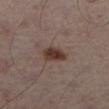Clinical impression: The lesion was photographed on a routine skin check and not biopsied; there is no pathology result. Clinical summary: A roughly 15 mm field-of-view crop from a total-body skin photograph. The lesion's longest dimension is about 3 mm. From the left leg. A male patient aged around 50. The total-body-photography lesion software estimated a lesion color around L≈35 a*≈16 b*≈23 in CIELAB, about 11 CIELAB-L* units darker than the surrounding skin, and a lesion-to-skin contrast of about 10.5 (normalized; higher = more distinct). The software also gave a nevus-likeness score of about 100/100 and a lesion-detection confidence of about 100/100. This is a cross-polarized tile.